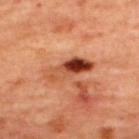Impression: Imaged during a routine full-body skin examination; the lesion was not biopsied and no histopathology is available.